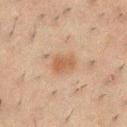follow-up=catalogued during a skin exam; not biopsied | lighting=cross-polarized illumination | patient=male, aged 48 to 52 | image-analysis metrics=a symmetry-axis asymmetry near 0.2; a border-irregularity index near 2/10, a color-variation rating of about 2/10, and a peripheral color-asymmetry measure near 1 | image source=total-body-photography crop, ~15 mm field of view | anatomic site=the chest | diameter=~3 mm (longest diameter).